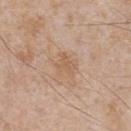Part of a total-body skin-imaging series; this lesion was reviewed on a skin check and was not flagged for biopsy. A male subject, in their mid- to late 60s. Located on the chest. Captured under white-light illumination. A 15 mm crop from a total-body photograph taken for skin-cancer surveillance.